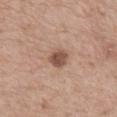biopsy_status: not biopsied; imaged during a skin examination
lighting: white-light
image:
  source: total-body photography crop
  field_of_view_mm: 15
lesion_size:
  long_diameter_mm_approx: 2.5
patient:
  sex: female
  age_approx: 65
site: mid back